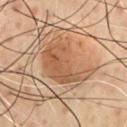| key | value |
|---|---|
| workup | imaged on a skin check; not biopsied |
| image source | 15 mm crop, total-body photography |
| subject | male, aged 68–72 |
| size | about 6.5 mm |
| location | the chest |
| tile lighting | cross-polarized illumination |